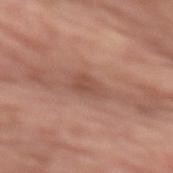Part of a total-body skin-imaging series; this lesion was reviewed on a skin check and was not flagged for biopsy.
From the lower back.
Imaged with white-light lighting.
The subject is a male roughly 70 years of age.
A 15 mm crop from a total-body photograph taken for skin-cancer surveillance.
Automated tile analysis of the lesion measured about 9 CIELAB-L* units darker than the surrounding skin. The analysis additionally found a border-irregularity index near 3/10 and peripheral color asymmetry of about 1.